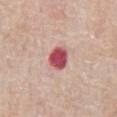| field | value |
|---|---|
| notes | no biopsy performed (imaged during a skin exam) |
| image source | ~15 mm crop, total-body skin-cancer survey |
| patient | male, in their mid-60s |
| location | the front of the torso |
| automated lesion analysis | a lesion-to-skin contrast of about 12.5 (normalized; higher = more distinct); border irregularity of about 1.5 on a 0–10 scale, internal color variation of about 3 on a 0–10 scale, and a peripheral color-asymmetry measure near 1 |